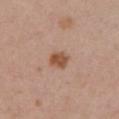{"biopsy_status": "not biopsied; imaged during a skin examination", "automated_metrics": {"border_irregularity_0_10": 1.5, "color_variation_0_10": 2.0, "peripheral_color_asymmetry": 0.5, "nevus_likeness_0_100": 95, "lesion_detection_confidence_0_100": 100}, "site": "chest", "lesion_size": {"long_diameter_mm_approx": 2.5}, "lighting": "white-light", "image": {"source": "total-body photography crop", "field_of_view_mm": 15}, "patient": {"sex": "female", "age_approx": 55}}